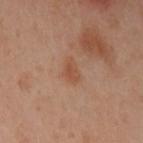workup = no biopsy performed (imaged during a skin exam) | subject = female, in their 40s | site = the left upper arm | image = total-body-photography crop, ~15 mm field of view.